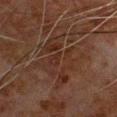Q: Was a biopsy performed?
A: imaged on a skin check; not biopsied
Q: Lesion location?
A: the front of the torso
Q: Lesion size?
A: ≈8.5 mm
Q: How was the tile lit?
A: cross-polarized illumination
Q: What kind of image is this?
A: 15 mm crop, total-body photography
Q: Automated lesion metrics?
A: a mean CIELAB color near L≈23 a*≈15 b*≈21, about 4 CIELAB-L* units darker than the surrounding skin, and a normalized border contrast of about 4.5; a classifier nevus-likeness of about 0/100 and a detector confidence of about 55 out of 100 that the crop contains a lesion
Q: Who is the patient?
A: male, approximately 80 years of age